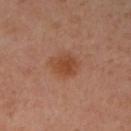{
  "biopsy_status": "not biopsied; imaged during a skin examination",
  "lesion_size": {
    "long_diameter_mm_approx": 3.5
  },
  "automated_metrics": {
    "cielab_L": 46,
    "cielab_a": 24,
    "cielab_b": 33,
    "vs_skin_darker_L": 8.0,
    "nevus_likeness_0_100": 90
  },
  "site": "left arm",
  "image": {
    "source": "total-body photography crop",
    "field_of_view_mm": 15
  },
  "patient": {
    "sex": "female",
    "age_approx": 40
  }
}